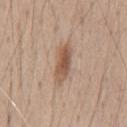Located on the chest. Longest diameter approximately 5 mm. The patient is a male aged around 45. The tile uses white-light illumination. A roughly 15 mm field-of-view crop from a total-body skin photograph. Automated image analysis of the tile measured an eccentricity of roughly 0.9 and a shape-asymmetry score of about 0.15 (0 = symmetric). The software also gave an automated nevus-likeness rating near 100 out of 100.